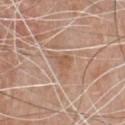<record>
<biopsy_status>not biopsied; imaged during a skin examination</biopsy_status>
</record>